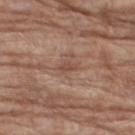Imaged during a routine full-body skin examination; the lesion was not biopsied and no histopathology is available.
A female patient aged 73 to 77.
A 15 mm close-up tile from a total-body photography series done for melanoma screening.
The lesion's longest dimension is about 2.5 mm.
From the right thigh.
Captured under white-light illumination.
The total-body-photography lesion software estimated an eccentricity of roughly 0.85. And it measured a lesion–skin lightness drop of about 8 and a normalized border contrast of about 6. The analysis additionally found a classifier nevus-likeness of about 0/100 and lesion-presence confidence of about 95/100.